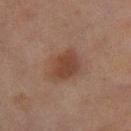Clinical impression: No biopsy was performed on this lesion — it was imaged during a full skin examination and was not determined to be concerning. Acquisition and patient details: The recorded lesion diameter is about 4 mm. Cropped from a total-body skin-imaging series; the visible field is about 15 mm. The patient is a female aged around 60. From the right lower leg. This is a cross-polarized tile.